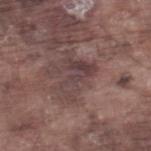This lesion was catalogued during total-body skin photography and was not selected for biopsy. The subject is a male aged around 75. From the right lower leg. About 5 mm across. A 15 mm close-up tile from a total-body photography series done for melanoma screening. The total-body-photography lesion software estimated a lesion area of about 10 mm², an outline eccentricity of about 0.75 (0 = round, 1 = elongated), and a symmetry-axis asymmetry near 0.6. The software also gave an average lesion color of about L≈41 a*≈17 b*≈17 (CIELAB). Imaged with white-light lighting.